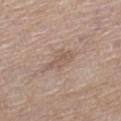biopsy status = catalogued during a skin exam; not biopsied | site = the left thigh | subject = female, about 65 years old | image = total-body-photography crop, ~15 mm field of view | diameter = ≈4 mm | lighting = white-light.